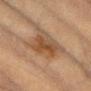The lesion was photographed on a routine skin check and not biopsied; there is no pathology result. The patient is a female roughly 80 years of age. The lesion is located on the chest. This image is a 15 mm lesion crop taken from a total-body photograph.